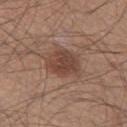Findings:
– notes — no biopsy performed (imaged during a skin exam)
– lighting — white-light illumination
– subject — male, in their mid- to late 40s
– automated metrics — a lesion color around L≈43 a*≈19 b*≈26 in CIELAB and roughly 10 lightness units darker than nearby skin; a border-irregularity index near 1.5/10, internal color variation of about 3 on a 0–10 scale, and peripheral color asymmetry of about 1
– lesion size — ≈4 mm
– image source — total-body-photography crop, ~15 mm field of view
– location — the left thigh